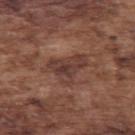follow-up: imaged on a skin check; not biopsied
acquisition: ~15 mm crop, total-body skin-cancer survey
lesion diameter: ≈4.5 mm
subject: male, in their mid-70s
illumination: white-light illumination
body site: the arm